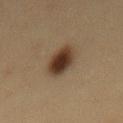Assessment:
Part of a total-body skin-imaging series; this lesion was reviewed on a skin check and was not flagged for biopsy.
Acquisition and patient details:
A female subject about 50 years old. A 15 mm crop from a total-body photograph taken for skin-cancer surveillance. The lesion is located on the mid back. Longest diameter approximately 4.5 mm.